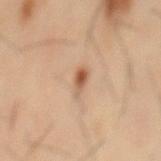Notes:
* notes: imaged on a skin check; not biopsied
* acquisition: 15 mm crop, total-body photography
* site: the mid back
* patient: male, approximately 40 years of age